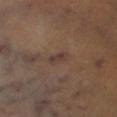{
  "site": "right lower leg",
  "image": {
    "source": "total-body photography crop",
    "field_of_view_mm": 15
  },
  "lighting": "cross-polarized",
  "automated_metrics": {
    "area_mm2_approx": 3.0,
    "eccentricity": 0.9,
    "shape_asymmetry": 0.2
  },
  "patient": {
    "sex": "male",
    "age_approx": 50
  },
  "lesion_size": {
    "long_diameter_mm_approx": 2.5
  }
}